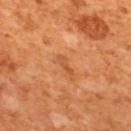| key | value |
|---|---|
| workup | no biopsy performed (imaged during a skin exam) |
| acquisition | total-body-photography crop, ~15 mm field of view |
| anatomic site | the upper back |
| automated metrics | a nevus-likeness score of about 10/100 and lesion-presence confidence of about 100/100 |
| tile lighting | cross-polarized |
| diameter | about 2.5 mm |
| patient | male, aged approximately 65 |